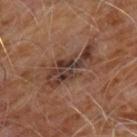biopsy status — imaged on a skin check; not biopsied | illumination — cross-polarized illumination | anatomic site — the chest | lesion size — ≈6.5 mm | acquisition — ~15 mm crop, total-body skin-cancer survey | subject — male, aged 58–62.